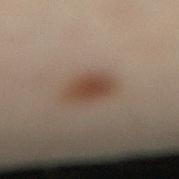No biopsy was performed on this lesion — it was imaged during a full skin examination and was not determined to be concerning. From the leg. A 15 mm close-up tile from a total-body photography series done for melanoma screening. Imaged with cross-polarized lighting. The patient is a male aged 48 to 52. The recorded lesion diameter is about 3.5 mm.